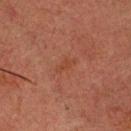Impression: Captured during whole-body skin photography for melanoma surveillance; the lesion was not biopsied. Acquisition and patient details: Imaged with cross-polarized lighting. Automated tile analysis of the lesion measured an average lesion color of about L≈33 a*≈20 b*≈26 (CIELAB), roughly 4 lightness units darker than nearby skin, and a normalized lesion–skin contrast near 4.5. It also reported a classifier nevus-likeness of about 0/100. The lesion is located on the head or neck. A 15 mm crop from a total-body photograph taken for skin-cancer surveillance. A male subject, in their 60s. The recorded lesion diameter is about 3 mm.